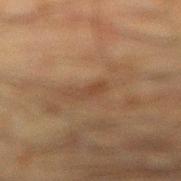Q: Was this lesion biopsied?
A: catalogued during a skin exam; not biopsied
Q: How was the tile lit?
A: cross-polarized
Q: What kind of image is this?
A: 15 mm crop, total-body photography
Q: Who is the patient?
A: male, aged approximately 35
Q: Lesion location?
A: the right lower leg